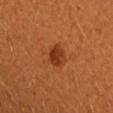Assessment: Part of a total-body skin-imaging series; this lesion was reviewed on a skin check and was not flagged for biopsy. Background: Cropped from a whole-body photographic skin survey; the tile spans about 15 mm. A female patient, about 30 years old. From the right forearm. Measured at roughly 2.5 mm in maximum diameter. Captured under cross-polarized illumination.